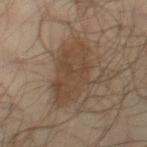<case>
  <biopsy_status>not biopsied; imaged during a skin examination</biopsy_status>
  <lighting>cross-polarized</lighting>
  <site>right thigh</site>
  <patient>
    <sex>male</sex>
    <age_approx>65</age_approx>
  </patient>
  <automated_metrics>
    <area_mm2_approx>23.0</area_mm2_approx>
    <eccentricity>0.6</eccentricity>
    <shape_asymmetry>0.3</shape_asymmetry>
    <lesion_detection_confidence_0_100>100</lesion_detection_confidence_0_100>
  </automated_metrics>
  <image>
    <source>total-body photography crop</source>
    <field_of_view_mm>15</field_of_view_mm>
  </image>
  <lesion_size>
    <long_diameter_mm_approx>6.0</long_diameter_mm_approx>
  </lesion_size>
</case>